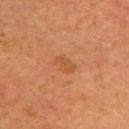follow-up: total-body-photography surveillance lesion; no biopsy
acquisition: total-body-photography crop, ~15 mm field of view
diameter: about 3 mm
image-analysis metrics: an area of roughly 4.5 mm²
location: the upper back
subject: female, in their mid-50s
tile lighting: cross-polarized illumination